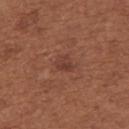follow-up: total-body-photography surveillance lesion; no biopsy | image source: ~15 mm tile from a whole-body skin photo | image-analysis metrics: a footprint of about 3.5 mm², an outline eccentricity of about 0.7 (0 = round, 1 = elongated), and two-axis asymmetry of about 0.3; roughly 7 lightness units darker than nearby skin and a lesion-to-skin contrast of about 6 (normalized; higher = more distinct); a detector confidence of about 100 out of 100 that the crop contains a lesion | anatomic site: the upper back | lighting: white-light | patient: female, about 65 years old.